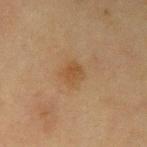Notes:
* biopsy status — imaged on a skin check; not biopsied
* lesion size — ≈2.5 mm
* lighting — cross-polarized
* patient — male, approximately 65 years of age
* anatomic site — the arm
* imaging modality — total-body-photography crop, ~15 mm field of view